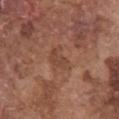subject: male, in their mid- to late 70s | anatomic site: the chest | tile lighting: white-light illumination | diameter: about 3.5 mm | automated metrics: a footprint of about 5.5 mm², an eccentricity of roughly 0.75, and two-axis asymmetry of about 0.45; a lesion-to-skin contrast of about 4.5 (normalized; higher = more distinct); border irregularity of about 4.5 on a 0–10 scale and a peripheral color-asymmetry measure near 0.5 | acquisition: ~15 mm tile from a whole-body skin photo.